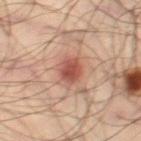{"biopsy_status": "not biopsied; imaged during a skin examination", "patient": {"sex": "male", "age_approx": 35}, "lighting": "cross-polarized", "lesion_size": {"long_diameter_mm_approx": 4.0}, "site": "right thigh", "image": {"source": "total-body photography crop", "field_of_view_mm": 15}}